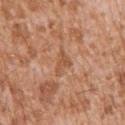follow-up: imaged on a skin check; not biopsied | subject: male, in their mid- to late 40s | lesion size: ≈3 mm | image: ~15 mm tile from a whole-body skin photo | body site: the left upper arm.